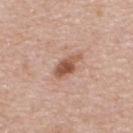The lesion was photographed on a routine skin check and not biopsied; there is no pathology result.
A 15 mm close-up extracted from a 3D total-body photography capture.
A female patient, approximately 45 years of age.
The lesion is located on the upper back.